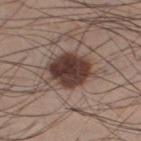Captured during whole-body skin photography for melanoma surveillance; the lesion was not biopsied.
A 15 mm close-up tile from a total-body photography series done for melanoma screening.
A male patient in their mid- to late 30s.
Approximately 5 mm at its widest.
The lesion-visualizer software estimated a lesion area of about 14 mm² and a shape-asymmetry score of about 0.15 (0 = symmetric). And it measured lesion-presence confidence of about 100/100.
Located on the right thigh.